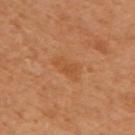{
  "biopsy_status": "not biopsied; imaged during a skin examination",
  "lesion_size": {
    "long_diameter_mm_approx": 3.5
  },
  "automated_metrics": {
    "eccentricity": 0.7,
    "cielab_L": 44,
    "cielab_a": 21,
    "cielab_b": 35,
    "vs_skin_darker_L": 5.0,
    "vs_skin_contrast_norm": 5.0,
    "border_irregularity_0_10": 2.5,
    "color_variation_0_10": 2.0,
    "peripheral_color_asymmetry": 0.5,
    "nevus_likeness_0_100": 5
  },
  "site": "arm",
  "image": {
    "source": "total-body photography crop",
    "field_of_view_mm": 15
  },
  "lighting": "cross-polarized",
  "patient": {
    "sex": "female",
    "age_approx": 60
  }
}